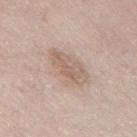Captured during whole-body skin photography for melanoma surveillance; the lesion was not biopsied. The lesion's longest dimension is about 5 mm. A roughly 15 mm field-of-view crop from a total-body skin photograph. A male subject, roughly 75 years of age. Located on the mid back. The tile uses white-light illumination.